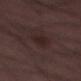This lesion was catalogued during total-body skin photography and was not selected for biopsy. From the left lower leg. The subject is a male roughly 50 years of age. The lesion's longest dimension is about 3 mm. A roughly 15 mm field-of-view crop from a total-body skin photograph. Imaged with white-light lighting. An algorithmic analysis of the crop reported a normalized lesion–skin contrast near 6.5. It also reported border irregularity of about 3 on a 0–10 scale, a color-variation rating of about 2.5/10, and peripheral color asymmetry of about 1.